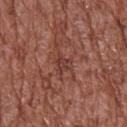notes: catalogued during a skin exam; not biopsied | image: ~15 mm tile from a whole-body skin photo | diameter: about 3 mm | location: the upper back | subject: male, aged approximately 75 | lighting: white-light | automated metrics: border irregularity of about 4.5 on a 0–10 scale and internal color variation of about 2 on a 0–10 scale.